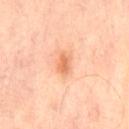Clinical impression:
Part of a total-body skin-imaging series; this lesion was reviewed on a skin check and was not flagged for biopsy.
Acquisition and patient details:
A 15 mm close-up extracted from a 3D total-body photography capture. A male subject aged 63–67. The lesion is on the lower back. The total-body-photography lesion software estimated a lesion area of about 3.5 mm², an outline eccentricity of about 0.75 (0 = round, 1 = elongated), and a shape-asymmetry score of about 0.3 (0 = symmetric).